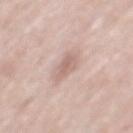The lesion was tiled from a total-body skin photograph and was not biopsied. Cropped from a total-body skin-imaging series; the visible field is about 15 mm. A male subject, roughly 55 years of age. On the mid back.